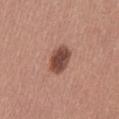Findings:
- notes · imaged on a skin check; not biopsied
- image · 15 mm crop, total-body photography
- lesion diameter · about 3.5 mm
- location · the front of the torso
- subject · female, aged 48 to 52
- tile lighting · white-light
- image-analysis metrics · an area of roughly 7.5 mm² and a shape-asymmetry score of about 0.2 (0 = symmetric); an average lesion color of about L≈46 a*≈23 b*≈26 (CIELAB), roughly 15 lightness units darker than nearby skin, and a normalized border contrast of about 11; a border-irregularity rating of about 1.5/10 and peripheral color asymmetry of about 1; a classifier nevus-likeness of about 60/100 and a lesion-detection confidence of about 100/100